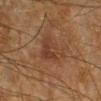Clinical impression:
Captured during whole-body skin photography for melanoma surveillance; the lesion was not biopsied.
Context:
The patient is a male about 65 years old. From the leg. A 15 mm close-up tile from a total-body photography series done for melanoma screening. Captured under cross-polarized illumination.